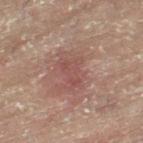workup — total-body-photography surveillance lesion; no biopsy | image source — ~15 mm tile from a whole-body skin photo | patient — female, in their 80s | location — the right leg.